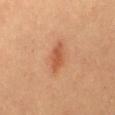No biopsy was performed on this lesion — it was imaged during a full skin examination and was not determined to be concerning. An algorithmic analysis of the crop reported border irregularity of about 2.5 on a 0–10 scale, internal color variation of about 2 on a 0–10 scale, and a peripheral color-asymmetry measure near 1. Captured under cross-polarized illumination. A female patient, aged 58 to 62. On the mid back. Longest diameter approximately 4 mm. This image is a 15 mm lesion crop taken from a total-body photograph.